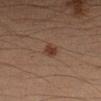Imaged during a routine full-body skin examination; the lesion was not biopsied and no histopathology is available. This image is a 15 mm lesion crop taken from a total-body photograph. On the arm. Captured under cross-polarized illumination. The total-body-photography lesion software estimated a lesion area of about 3.5 mm², an eccentricity of roughly 0.7, and a symmetry-axis asymmetry near 0.25. The software also gave a border-irregularity index near 2.5/10, a within-lesion color-variation index near 1.5/10, and radial color variation of about 0.5. The analysis additionally found a nevus-likeness score of about 95/100 and a lesion-detection confidence of about 100/100. Longest diameter approximately 2.5 mm. A male subject roughly 35 years of age.